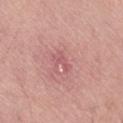Recorded during total-body skin imaging; not selected for excision or biopsy.
The recorded lesion diameter is about 3 mm.
A male patient aged 53 to 57.
Cropped from a total-body skin-imaging series; the visible field is about 15 mm.
Captured under white-light illumination.
The lesion is on the lower back.